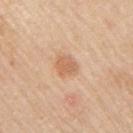Impression:
Imaged during a routine full-body skin examination; the lesion was not biopsied and no histopathology is available.